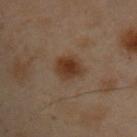Notes:
- notes · catalogued during a skin exam; not biopsied
- tile lighting · cross-polarized illumination
- image · 15 mm crop, total-body photography
- location · the chest
- image-analysis metrics · an average lesion color of about L≈28 a*≈14 b*≈24 (CIELAB) and a lesion–skin lightness drop of about 8
- subject · male, aged approximately 55